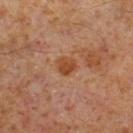Findings:
* follow-up: catalogued during a skin exam; not biopsied
* subject: male, aged approximately 60
* site: the right lower leg
* image-analysis metrics: a footprint of about 4.5 mm², an eccentricity of roughly 0.5, and a symmetry-axis asymmetry near 0.2; a lesion color around L≈43 a*≈23 b*≈34 in CIELAB, about 8 CIELAB-L* units darker than the surrounding skin, and a normalized lesion–skin contrast near 8
* imaging modality: total-body-photography crop, ~15 mm field of view
* tile lighting: cross-polarized illumination
* diameter: ≈2.5 mm